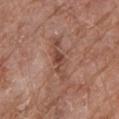<record>
<biopsy_status>not biopsied; imaged during a skin examination</biopsy_status>
<image>
  <source>total-body photography crop</source>
  <field_of_view_mm>15</field_of_view_mm>
</image>
<patient>
  <sex>male</sex>
  <age_approx>80</age_approx>
</patient>
<automated_metrics>
  <cielab_L>46</cielab_L>
  <cielab_a>22</cielab_a>
  <cielab_b>27</cielab_b>
  <vs_skin_darker_L>9.0</vs_skin_darker_L>
</automated_metrics>
<site>right forearm</site>
<lesion_size>
  <long_diameter_mm_approx>5.0</long_diameter_mm_approx>
</lesion_size>
</record>